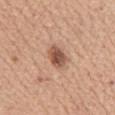– workup · no biopsy performed (imaged during a skin exam)
– subject · female, in their mid- to late 50s
– tile lighting · white-light
– site · the abdomen
– image-analysis metrics · a footprint of about 5.5 mm², an outline eccentricity of about 0.75 (0 = round, 1 = elongated), and two-axis asymmetry of about 0.2; roughly 14 lightness units darker than nearby skin and a lesion-to-skin contrast of about 9.5 (normalized; higher = more distinct); a border-irregularity index near 1.5/10, internal color variation of about 4.5 on a 0–10 scale, and peripheral color asymmetry of about 1.5
– image source · 15 mm crop, total-body photography
– size · ~3 mm (longest diameter)